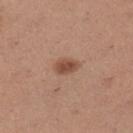biopsy status: imaged on a skin check; not biopsied
automated lesion analysis: a border-irregularity index near 2/10 and a color-variation rating of about 2/10; an automated nevus-likeness rating near 95 out of 100 and a detector confidence of about 100 out of 100 that the crop contains a lesion
subject: female, about 30 years old
illumination: white-light illumination
lesion size: ~3 mm (longest diameter)
acquisition: ~15 mm tile from a whole-body skin photo
body site: the left lower leg